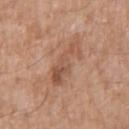workup=imaged on a skin check; not biopsied
subject=male, aged approximately 60
anatomic site=the right upper arm
imaging modality=15 mm crop, total-body photography